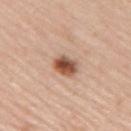Recorded during total-body skin imaging; not selected for excision or biopsy.
A male subject, aged 43 to 47.
On the back.
Imaged with white-light lighting.
Measured at roughly 3.5 mm in maximum diameter.
A 15 mm close-up tile from a total-body photography series done for melanoma screening.